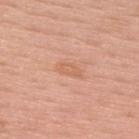Impression:
Part of a total-body skin-imaging series; this lesion was reviewed on a skin check and was not flagged for biopsy.
Context:
Located on the upper back. The patient is a male aged around 55. The recorded lesion diameter is about 3 mm. A roughly 15 mm field-of-view crop from a total-body skin photograph.